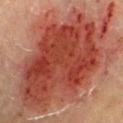Q: Was a biopsy performed?
A: catalogued during a skin exam; not biopsied
Q: Patient demographics?
A: male, in their mid-80s or older
Q: How was the tile lit?
A: cross-polarized illumination
Q: What is the lesion's diameter?
A: ~17.5 mm (longest diameter)
Q: How was this image acquired?
A: total-body-photography crop, ~15 mm field of view
Q: What is the anatomic site?
A: the right lower leg